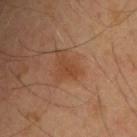The lesion was tiled from a total-body skin photograph and was not biopsied. An algorithmic analysis of the crop reported roughly 6 lightness units darker than nearby skin and a normalized border contrast of about 5.5. The analysis additionally found an automated nevus-likeness rating near 0 out of 100 and lesion-presence confidence of about 100/100. A male patient aged around 55. Cropped from a total-body skin-imaging series; the visible field is about 15 mm. The lesion is located on the upper back. Captured under cross-polarized illumination.